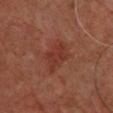follow-up: imaged on a skin check; not biopsied | body site: the upper back | image source: 15 mm crop, total-body photography | subject: male, roughly 60 years of age | size: ≈4.5 mm | automated lesion analysis: a footprint of about 9.5 mm², an outline eccentricity of about 0.8 (0 = round, 1 = elongated), and a symmetry-axis asymmetry near 0.3; a mean CIELAB color near L≈31 a*≈24 b*≈25 and roughly 6 lightness units darker than nearby skin; a detector confidence of about 100 out of 100 that the crop contains a lesion.